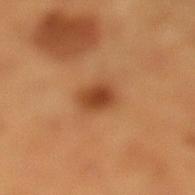Part of a total-body skin-imaging series; this lesion was reviewed on a skin check and was not flagged for biopsy.
From the left lower leg.
This image is a 15 mm lesion crop taken from a total-body photograph.
Longest diameter approximately 3 mm.
The tile uses cross-polarized illumination.
A male subject in their mid- to late 50s.